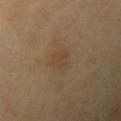No biopsy was performed on this lesion — it was imaged during a full skin examination and was not determined to be concerning.
The patient is a male about 40 years old.
On the arm.
A 15 mm crop from a total-body photograph taken for skin-cancer surveillance.